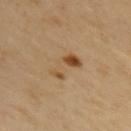notes: total-body-photography surveillance lesion; no biopsy